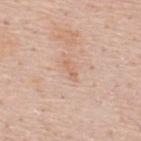biopsy status = imaged on a skin check; not biopsied
site = the upper back
subject = male, about 50 years old
imaging modality = ~15 mm crop, total-body skin-cancer survey
lesion size = about 2.5 mm
lighting = white-light